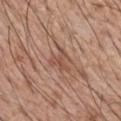• follow-up: imaged on a skin check; not biopsied
• image-analysis metrics: border irregularity of about 8 on a 0–10 scale, a color-variation rating of about 0/10, and a peripheral color-asymmetry measure near 0; a classifier nevus-likeness of about 0/100 and lesion-presence confidence of about 95/100
• diameter: ≈3 mm
• subject: male, in their mid- to late 50s
• illumination: white-light illumination
• acquisition: ~15 mm tile from a whole-body skin photo
• location: the right upper arm